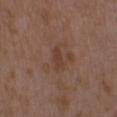biopsy_status: not biopsied; imaged during a skin examination
lighting: white-light
site: upper back
automated_metrics:
  area_mm2_approx: 3.0
  eccentricity: 0.9
  shape_asymmetry: 0.3
  border_irregularity_0_10: 3.5
  color_variation_0_10: 0.5
  peripheral_color_asymmetry: 0.0
lesion_size:
  long_diameter_mm_approx: 3.0
image:
  source: total-body photography crop
  field_of_view_mm: 15
patient:
  sex: female
  age_approx: 30